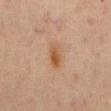Q: Was this lesion biopsied?
A: imaged on a skin check; not biopsied
Q: What kind of image is this?
A: 15 mm crop, total-body photography
Q: How large is the lesion?
A: about 3.5 mm
Q: Who is the patient?
A: male, in their 70s
Q: Lesion location?
A: the abdomen
Q: Illumination type?
A: cross-polarized illumination